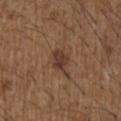Case summary:
* biopsy status — catalogued during a skin exam; not biopsied
* subject — male, aged approximately 50
* body site — the right upper arm
* illumination — white-light
* image-analysis metrics — an eccentricity of roughly 0.65; a lesion–skin lightness drop of about 9 and a lesion-to-skin contrast of about 8 (normalized; higher = more distinct); a border-irregularity index near 2.5/10 and peripheral color asymmetry of about 1; an automated nevus-likeness rating near 60 out of 100
* size — ≈3 mm
* image — total-body-photography crop, ~15 mm field of view